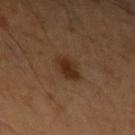  patient:
    sex: male
    age_approx: 65
  image:
    source: total-body photography crop
    field_of_view_mm: 15
  lesion_size:
    long_diameter_mm_approx: 3.5
  lighting: cross-polarized
  automated_metrics:
    area_mm2_approx: 6.5
    eccentricity: 0.65
    shape_asymmetry: 0.15
  site: left upper arm